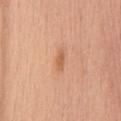<case>
  <biopsy_status>not biopsied; imaged during a skin examination</biopsy_status>
  <lighting>white-light</lighting>
  <site>mid back</site>
  <automated_metrics>
    <eccentricity>0.9</eccentricity>
    <shape_asymmetry>0.35</shape_asymmetry>
    <cielab_L>61</cielab_L>
    <cielab_a>25</cielab_a>
    <cielab_b>37</cielab_b>
    <vs_skin_darker_L>9.0</vs_skin_darker_L>
    <vs_skin_contrast_norm>6.5</vs_skin_contrast_norm>
    <border_irregularity_0_10>3.5</border_irregularity_0_10>
    <peripheral_color_asymmetry>0.0</peripheral_color_asymmetry>
    <lesion_detection_confidence_0_100>100</lesion_detection_confidence_0_100>
  </automated_metrics>
  <lesion_size>
    <long_diameter_mm_approx>2.5</long_diameter_mm_approx>
  </lesion_size>
  <patient>
    <sex>female</sex>
    <age_approx>50</age_approx>
  </patient>
  <image>
    <source>total-body photography crop</source>
    <field_of_view_mm>15</field_of_view_mm>
  </image>
</case>